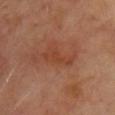workup=total-body-photography surveillance lesion; no biopsy
illumination=cross-polarized illumination
patient=male, about 65 years old
diameter=about 4 mm
site=the chest
image source=15 mm crop, total-body photography